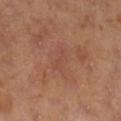Q: Is there a histopathology result?
A: imaged on a skin check; not biopsied
Q: What lighting was used for the tile?
A: cross-polarized illumination
Q: What is the lesion's diameter?
A: about 3.5 mm
Q: What is the anatomic site?
A: the right lower leg
Q: What kind of image is this?
A: ~15 mm tile from a whole-body skin photo
Q: Automated lesion metrics?
A: a lesion color around L≈49 a*≈24 b*≈30 in CIELAB, roughly 4 lightness units darker than nearby skin, and a normalized lesion–skin contrast near 3
Q: What are the patient's age and sex?
A: female, aged around 50